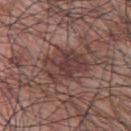<case>
<biopsy_status>not biopsied; imaged during a skin examination</biopsy_status>
<image>
  <source>total-body photography crop</source>
  <field_of_view_mm>15</field_of_view_mm>
</image>
<automated_metrics>
  <cielab_L>39</cielab_L>
  <cielab_a>19</cielab_a>
  <cielab_b>21</cielab_b>
  <vs_skin_contrast_norm>7.5</vs_skin_contrast_norm>
  <color_variation_0_10>4.5</color_variation_0_10>
  <peripheral_color_asymmetry>1.5</peripheral_color_asymmetry>
</automated_metrics>
<patient>
  <sex>male</sex>
  <age_approx>70</age_approx>
</patient>
<lighting>white-light</lighting>
<lesion_size>
  <long_diameter_mm_approx>5.5</long_diameter_mm_approx>
</lesion_size>
<site>upper back</site>
</case>